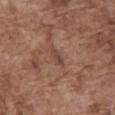Imaged during a routine full-body skin examination; the lesion was not biopsied and no histopathology is available. From the front of the torso. A male patient, aged 73 to 77. About 2.5 mm across. A roughly 15 mm field-of-view crop from a total-body skin photograph. This is a white-light tile.